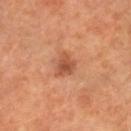Image and clinical context: A subject in their 70s. On the leg. Cropped from a whole-body photographic skin survey; the tile spans about 15 mm.